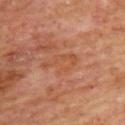No biopsy was performed on this lesion — it was imaged during a full skin examination and was not determined to be concerning. The tile uses cross-polarized illumination. Approximately 5 mm at its widest. The lesion-visualizer software estimated an area of roughly 6.5 mm², a shape eccentricity near 0.9, and a symmetry-axis asymmetry near 0.5. The analysis additionally found a lesion color around L≈50 a*≈25 b*≈35 in CIELAB, roughly 4 lightness units darker than nearby skin, and a lesion-to-skin contrast of about 4.5 (normalized; higher = more distinct). The software also gave border irregularity of about 6.5 on a 0–10 scale. The subject is a male about 70 years old. A region of skin cropped from a whole-body photographic capture, roughly 15 mm wide. The lesion is located on the upper back.